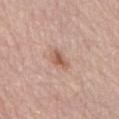* workup · no biopsy performed (imaged during a skin exam)